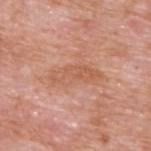Clinical summary:
The subject is a male approximately 60 years of age. Located on the upper back. A close-up tile cropped from a whole-body skin photograph, about 15 mm across.